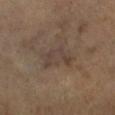| field | value |
|---|---|
| biopsy status | no biopsy performed (imaged during a skin exam) |
| size | ~4.5 mm (longest diameter) |
| image source | ~15 mm crop, total-body skin-cancer survey |
| patient | male, aged around 65 |